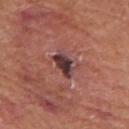Captured during whole-body skin photography for melanoma surveillance; the lesion was not biopsied. Measured at roughly 3 mm in maximum diameter. Automated image analysis of the tile measured a lesion area of about 5 mm² and an outline eccentricity of about 0.7 (0 = round, 1 = elongated). It also reported about 15 CIELAB-L* units darker than the surrounding skin and a normalized border contrast of about 13.5. The software also gave border irregularity of about 3.5 on a 0–10 scale, a color-variation rating of about 6/10, and peripheral color asymmetry of about 2. The analysis additionally found an automated nevus-likeness rating near 0 out of 100 and a detector confidence of about 100 out of 100 that the crop contains a lesion. A male subject aged approximately 80. Captured under white-light illumination. On the left upper arm. A roughly 15 mm field-of-view crop from a total-body skin photograph.